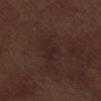Impression: This lesion was catalogued during total-body skin photography and was not selected for biopsy. Clinical summary: Imaged with white-light lighting. Measured at roughly 2.5 mm in maximum diameter. A region of skin cropped from a whole-body photographic capture, roughly 15 mm wide. A male subject in their 70s. From the leg.